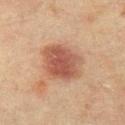biopsy_status: not biopsied; imaged during a skin examination
site: left thigh
automated_metrics:
  cielab_L: 47
  cielab_a: 22
  cielab_b: 28
  vs_skin_darker_L: 11.0
  vs_skin_contrast_norm: 8.5
  nevus_likeness_0_100: 100
  lesion_detection_confidence_0_100: 100
image:
  source: total-body photography crop
  field_of_view_mm: 15
lighting: cross-polarized
patient:
  sex: female
  age_approx: 55
lesion_size:
  long_diameter_mm_approx: 5.5